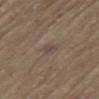Impression: Captured during whole-body skin photography for melanoma surveillance; the lesion was not biopsied. Clinical summary: The lesion is located on the upper back. The patient is a female approximately 80 years of age. Automated tile analysis of the lesion measured an area of roughly 3 mm², an outline eccentricity of about 0.85 (0 = round, 1 = elongated), and two-axis asymmetry of about 0.25. It also reported a lesion–skin lightness drop of about 5 and a normalized border contrast of about 6. The software also gave internal color variation of about 1 on a 0–10 scale and peripheral color asymmetry of about 0.5. It also reported an automated nevus-likeness rating near 0 out of 100 and a detector confidence of about 100 out of 100 that the crop contains a lesion. This is a cross-polarized tile. A lesion tile, about 15 mm wide, cut from a 3D total-body photograph. Approximately 2.5 mm at its widest.